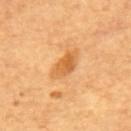Q: Was this lesion biopsied?
A: no biopsy performed (imaged during a skin exam)
Q: Illumination type?
A: cross-polarized illumination
Q: Lesion size?
A: ≈4.5 mm
Q: What is the imaging modality?
A: total-body-photography crop, ~15 mm field of view
Q: What is the anatomic site?
A: the upper back
Q: What are the patient's age and sex?
A: male, in their mid- to late 60s